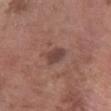<lesion>
  <biopsy_status>not biopsied; imaged during a skin examination</biopsy_status>
  <patient>
    <sex>male</sex>
    <age_approx>60</age_approx>
  </patient>
  <image>
    <source>total-body photography crop</source>
    <field_of_view_mm>15</field_of_view_mm>
  </image>
  <lesion_size>
    <long_diameter_mm_approx>2.5</long_diameter_mm_approx>
  </lesion_size>
  <site>right forearm</site>
</lesion>